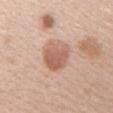Q: Was a biopsy performed?
A: no biopsy performed (imaged during a skin exam)
Q: What is the anatomic site?
A: the upper back
Q: How large is the lesion?
A: about 3.5 mm
Q: What are the patient's age and sex?
A: female, in their 40s
Q: Automated lesion metrics?
A: a lesion color around L≈60 a*≈22 b*≈29 in CIELAB, about 11 CIELAB-L* units darker than the surrounding skin, and a lesion-to-skin contrast of about 7.5 (normalized; higher = more distinct); a border-irregularity index near 2/10, a color-variation rating of about 4/10, and radial color variation of about 1.5; a lesion-detection confidence of about 100/100
Q: How was this image acquired?
A: ~15 mm tile from a whole-body skin photo